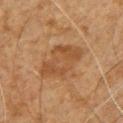Clinical impression:
Captured during whole-body skin photography for melanoma surveillance; the lesion was not biopsied.
Background:
The lesion is located on the front of the torso. Imaged with cross-polarized lighting. About 5.5 mm across. The subject is a male about 60 years old. A roughly 15 mm field-of-view crop from a total-body skin photograph.